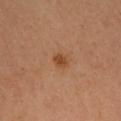{
  "image": {
    "source": "total-body photography crop",
    "field_of_view_mm": 15
  },
  "lesion_size": {
    "long_diameter_mm_approx": 2.0
  },
  "site": "head or neck",
  "patient": {
    "sex": "female",
    "age_approx": 35
  },
  "lighting": "cross-polarized",
  "automated_metrics": {
    "eccentricity": 0.35,
    "nevus_likeness_0_100": 80
  }
}